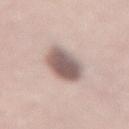{"biopsy_status": "not biopsied; imaged during a skin examination", "patient": {"sex": "female", "age_approx": 55}, "image": {"source": "total-body photography crop", "field_of_view_mm": 15}, "site": "lower back", "lesion_size": {"long_diameter_mm_approx": 5.0}}